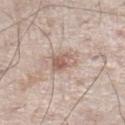Impression:
Imaged during a routine full-body skin examination; the lesion was not biopsied and no histopathology is available.
Background:
Longest diameter approximately 3 mm. Located on the leg. Imaged with white-light lighting. The lesion-visualizer software estimated a nevus-likeness score of about 15/100 and a lesion-detection confidence of about 100/100. A male subject aged 58–62. A roughly 15 mm field-of-view crop from a total-body skin photograph.